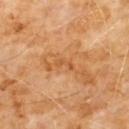This lesion was catalogued during total-body skin photography and was not selected for biopsy.
From the chest.
A male patient, approximately 60 years of age.
An algorithmic analysis of the crop reported a mean CIELAB color near L≈53 a*≈25 b*≈41 and a normalized border contrast of about 5.5. The analysis additionally found a classifier nevus-likeness of about 0/100 and lesion-presence confidence of about 95/100.
Cropped from a total-body skin-imaging series; the visible field is about 15 mm.
Imaged with cross-polarized lighting.